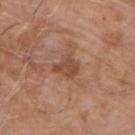Q: Was this lesion biopsied?
A: total-body-photography surveillance lesion; no biopsy
Q: What is the lesion's diameter?
A: ~3 mm (longest diameter)
Q: Where on the body is the lesion?
A: the upper back
Q: Who is the patient?
A: male, roughly 80 years of age
Q: How was the tile lit?
A: white-light illumination
Q: What did automated image analysis measure?
A: a lesion area of about 5.5 mm², an eccentricity of roughly 0.6, and a shape-asymmetry score of about 0.35 (0 = symmetric); a border-irregularity rating of about 3.5/10, internal color variation of about 2 on a 0–10 scale, and a peripheral color-asymmetry measure near 0.5; an automated nevus-likeness rating near 0 out of 100 and a lesion-detection confidence of about 100/100
Q: What is the imaging modality?
A: 15 mm crop, total-body photography